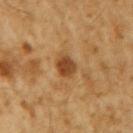Findings:
- workup · catalogued during a skin exam; not biopsied
- location · the right upper arm
- illumination · cross-polarized
- patient · male, aged approximately 60
- diameter · ~3 mm (longest diameter)
- TBP lesion metrics · a border-irregularity index near 2.5/10, a color-variation rating of about 2.5/10, and peripheral color asymmetry of about 1; lesion-presence confidence of about 100/100
- image source · 15 mm crop, total-body photography